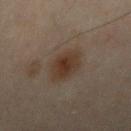<tbp_lesion>
  <biopsy_status>not biopsied; imaged during a skin examination</biopsy_status>
  <lighting>cross-polarized</lighting>
  <image>
    <source>total-body photography crop</source>
    <field_of_view_mm>15</field_of_view_mm>
  </image>
  <site>right upper arm</site>
  <patient>
    <sex>male</sex>
    <age_approx>50</age_approx>
  </patient>
</tbp_lesion>